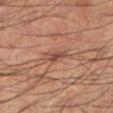The lesion is located on the left lower leg. This is a cross-polarized tile. A 15 mm close-up tile from a total-body photography series done for melanoma screening. The patient is a male aged around 60. The lesion-visualizer software estimated an area of roughly 3.5 mm², a shape eccentricity near 0.85, and a symmetry-axis asymmetry near 0.25. And it measured a nevus-likeness score of about 0/100 and lesion-presence confidence of about 95/100. Approximately 3 mm at its widest.